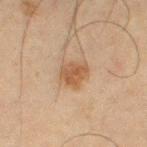Clinical impression: No biopsy was performed on this lesion — it was imaged during a full skin examination and was not determined to be concerning. Image and clinical context: A roughly 15 mm field-of-view crop from a total-body skin photograph. Imaged with cross-polarized lighting. From the left thigh. The subject is a male approximately 65 years of age.